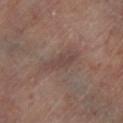Imaged during a routine full-body skin examination; the lesion was not biopsied and no histopathology is available. This is a cross-polarized tile. Approximately 4.5 mm at its widest. The lesion is on the left lower leg. A 15 mm crop from a total-body photograph taken for skin-cancer surveillance. The total-body-photography lesion software estimated a shape eccentricity near 0.9 and a symmetry-axis asymmetry near 0.25. It also reported a border-irregularity index near 3.5/10, internal color variation of about 2 on a 0–10 scale, and peripheral color asymmetry of about 0.5.